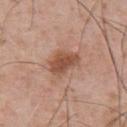Recorded during total-body skin imaging; not selected for excision or biopsy. Automated tile analysis of the lesion measured a lesion area of about 8.5 mm² and a shape eccentricity near 0.75. The analysis additionally found a lesion–skin lightness drop of about 12 and a lesion-to-skin contrast of about 8.5 (normalized; higher = more distinct). The analysis additionally found border irregularity of about 3 on a 0–10 scale, internal color variation of about 3 on a 0–10 scale, and radial color variation of about 1. A lesion tile, about 15 mm wide, cut from a 3D total-body photograph. The lesion is on the upper back. The patient is a male about 55 years old. The tile uses white-light illumination.